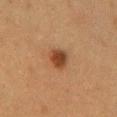Part of a total-body skin-imaging series; this lesion was reviewed on a skin check and was not flagged for biopsy.
A female subject about 50 years old.
A close-up tile cropped from a whole-body skin photograph, about 15 mm across.
Automated image analysis of the tile measured an outline eccentricity of about 0.6 (0 = round, 1 = elongated) and two-axis asymmetry of about 0.15. The software also gave a classifier nevus-likeness of about 100/100.
The lesion's longest dimension is about 2.5 mm.
On the chest.